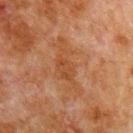<case>
  <biopsy_status>not biopsied; imaged during a skin examination</biopsy_status>
  <patient>
    <sex>male</sex>
    <age_approx>80</age_approx>
  </patient>
  <lesion_size>
    <long_diameter_mm_approx>7.0</long_diameter_mm_approx>
  </lesion_size>
  <site>chest</site>
  <automated_metrics>
    <cielab_L>38</cielab_L>
    <cielab_a>20</cielab_a>
    <cielab_b>31</cielab_b>
    <vs_skin_contrast_norm>6.0</vs_skin_contrast_norm>
  </automated_metrics>
  <lighting>cross-polarized</lighting>
  <image>
    <source>total-body photography crop</source>
    <field_of_view_mm>15</field_of_view_mm>
  </image>
</case>